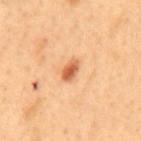<case>
<biopsy_status>not biopsied; imaged during a skin examination</biopsy_status>
<image>
  <source>total-body photography crop</source>
  <field_of_view_mm>15</field_of_view_mm>
</image>
<lighting>cross-polarized</lighting>
<site>mid back</site>
<lesion_size>
  <long_diameter_mm_approx>3.0</long_diameter_mm_approx>
</lesion_size>
<automated_metrics>
  <eccentricity>0.8</eccentricity>
  <shape_asymmetry>0.2</shape_asymmetry>
  <cielab_L>62</cielab_L>
  <cielab_a>29</cielab_a>
  <cielab_b>42</cielab_b>
  <vs_skin_darker_L>15.0</vs_skin_darker_L>
  <vs_skin_contrast_norm>9.0</vs_skin_contrast_norm>
  <color_variation_0_10>3.5</color_variation_0_10>
  <peripheral_color_asymmetry>1.0</peripheral_color_asymmetry>
</automated_metrics>
<patient>
  <sex>male</sex>
  <age_approx>50</age_approx>
</patient>
</case>